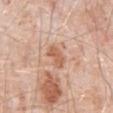Imaged during a routine full-body skin examination; the lesion was not biopsied and no histopathology is available. A region of skin cropped from a whole-body photographic capture, roughly 15 mm wide. Located on the abdomen. A male patient, roughly 80 years of age.